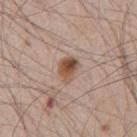automated metrics: a footprint of about 5.5 mm² and an outline eccentricity of about 0.65 (0 = round, 1 = elongated); a mean CIELAB color near L≈51 a*≈18 b*≈29 and a normalized border contrast of about 10; border irregularity of about 2 on a 0–10 scale, a within-lesion color-variation index near 7.5/10, and peripheral color asymmetry of about 3; a nevus-likeness score of about 95/100 and lesion-presence confidence of about 100/100
lesion diameter: about 3 mm
patient: male, aged 43 to 47
location: the chest
illumination: white-light
image source: 15 mm crop, total-body photography